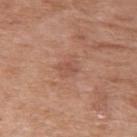Case summary:
- subject: male, aged 83 or over
- imaging modality: total-body-photography crop, ~15 mm field of view
- lesion size: ≈2.5 mm
- body site: the right upper arm